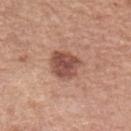biopsy_status: not biopsied; imaged during a skin examination
lesion_size:
  long_diameter_mm_approx: 3.5
patient:
  sex: female
  age_approx: 60
site: left forearm
image:
  source: total-body photography crop
  field_of_view_mm: 15
automated_metrics:
  area_mm2_approx: 9.5
  cielab_L: 50
  cielab_a: 23
  cielab_b: 27
  vs_skin_contrast_norm: 9.0
  nevus_likeness_0_100: 55